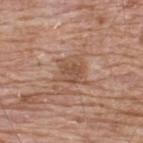The lesion was tiled from a total-body skin photograph and was not biopsied.
A 15 mm close-up extracted from a 3D total-body photography capture.
From the upper back.
The subject is a male approximately 65 years of age.